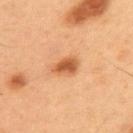Assessment: This lesion was catalogued during total-body skin photography and was not selected for biopsy. Background: The total-body-photography lesion software estimated a lesion area of about 5.5 mm², a shape eccentricity near 0.8, and a shape-asymmetry score of about 0.2 (0 = symmetric). And it measured a mean CIELAB color near L≈56 a*≈27 b*≈41, a lesion–skin lightness drop of about 13, and a lesion-to-skin contrast of about 8.5 (normalized; higher = more distinct). The software also gave a border-irregularity index near 2/10, internal color variation of about 3 on a 0–10 scale, and a peripheral color-asymmetry measure near 1. A 15 mm close-up tile from a total-body photography series done for melanoma screening. Imaged with cross-polarized lighting. A male patient aged 53–57. About 3.5 mm across. The lesion is located on the upper back.